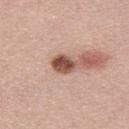Q: Was a biopsy performed?
A: no biopsy performed (imaged during a skin exam)
Q: What are the patient's age and sex?
A: male, aged 28–32
Q: Automated lesion metrics?
A: a footprint of about 5.5 mm² and two-axis asymmetry of about 0.15
Q: How large is the lesion?
A: ≈3.5 mm
Q: What is the imaging modality?
A: ~15 mm crop, total-body skin-cancer survey
Q: Illumination type?
A: white-light illumination